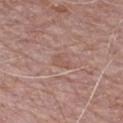{
  "biopsy_status": "not biopsied; imaged during a skin examination",
  "lesion_size": {
    "long_diameter_mm_approx": 2.5
  },
  "site": "chest",
  "lighting": "white-light",
  "image": {
    "source": "total-body photography crop",
    "field_of_view_mm": 15
  },
  "patient": {
    "sex": "male",
    "age_approx": 70
  }
}